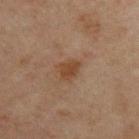Impression: Imaged during a routine full-body skin examination; the lesion was not biopsied and no histopathology is available. Background: A male patient in their mid- to late 40s. On the upper back. This is a cross-polarized tile. This image is a 15 mm lesion crop taken from a total-body photograph. Approximately 2.5 mm at its widest.